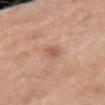Notes:
- notes · imaged on a skin check; not biopsied
- site · the arm
- image · ~15 mm tile from a whole-body skin photo
- patient · female, aged 53–57